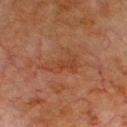notes = imaged on a skin check; not biopsied | patient = male, aged approximately 80 | imaging modality = 15 mm crop, total-body photography | body site = the chest | tile lighting = cross-polarized illumination | diameter = ≈5 mm.